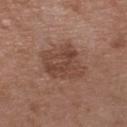{
  "biopsy_status": "not biopsied; imaged during a skin examination",
  "lighting": "white-light",
  "patient": {
    "sex": "male",
    "age_approx": 50
  },
  "lesion_size": {
    "long_diameter_mm_approx": 6.0
  },
  "automated_metrics": {
    "area_mm2_approx": 17.0,
    "eccentricity": 0.65,
    "shape_asymmetry": 0.15,
    "cielab_L": 44,
    "cielab_a": 20,
    "cielab_b": 26,
    "vs_skin_darker_L": 9.0,
    "vs_skin_contrast_norm": 7.0,
    "nevus_likeness_0_100": 10,
    "lesion_detection_confidence_0_100": 100
  },
  "site": "head or neck",
  "image": {
    "source": "total-body photography crop",
    "field_of_view_mm": 15
  }
}